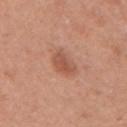The lesion was photographed on a routine skin check and not biopsied; there is no pathology result. The total-body-photography lesion software estimated an area of roughly 5.5 mm², an outline eccentricity of about 0.7 (0 = round, 1 = elongated), and two-axis asymmetry of about 0.3. The software also gave an average lesion color of about L≈53 a*≈25 b*≈31 (CIELAB), a lesion–skin lightness drop of about 9, and a normalized border contrast of about 6.5. It also reported a within-lesion color-variation index near 2.5/10 and radial color variation of about 1. A 15 mm crop from a total-body photograph taken for skin-cancer surveillance. Longest diameter approximately 3 mm. Located on the left upper arm. A female subject aged 33 to 37.